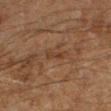| field | value |
|---|---|
| biopsy status | total-body-photography surveillance lesion; no biopsy |
| patient | male, roughly 60 years of age |
| acquisition | ~15 mm crop, total-body skin-cancer survey |
| TBP lesion metrics | about 5 CIELAB-L* units darker than the surrounding skin and a lesion-to-skin contrast of about 5.5 (normalized; higher = more distinct); border irregularity of about 3.5 on a 0–10 scale and internal color variation of about 0 on a 0–10 scale |
| anatomic site | the left lower leg |
| size | about 2.5 mm |